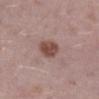Part of a total-body skin-imaging series; this lesion was reviewed on a skin check and was not flagged for biopsy. From the right lower leg. The tile uses white-light illumination. A 15 mm close-up tile from a total-body photography series done for melanoma screening. A male subject aged around 40. The total-body-photography lesion software estimated a lesion color around L≈45 a*≈21 b*≈23 in CIELAB, roughly 13 lightness units darker than nearby skin, and a normalized lesion–skin contrast near 10. The software also gave internal color variation of about 2 on a 0–10 scale. The analysis additionally found an automated nevus-likeness rating near 95 out of 100 and a lesion-detection confidence of about 100/100.